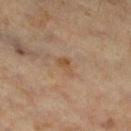Automated tile analysis of the lesion measured a shape eccentricity near 0.8 and two-axis asymmetry of about 0.4. The software also gave a mean CIELAB color near L≈47 a*≈16 b*≈31, about 6 CIELAB-L* units darker than the surrounding skin, and a lesion-to-skin contrast of about 6 (normalized; higher = more distinct).
The lesion is located on the right thigh.
The patient is a male about 60 years old.
Imaged with cross-polarized lighting.
This image is a 15 mm lesion crop taken from a total-body photograph.